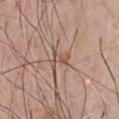The lesion was photographed on a routine skin check and not biopsied; there is no pathology result.
On the chest.
A male subject, aged approximately 50.
This image is a 15 mm lesion crop taken from a total-body photograph.